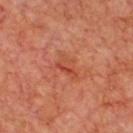The lesion was photographed on a routine skin check and not biopsied; there is no pathology result. Automated tile analysis of the lesion measured an area of roughly 4 mm², an outline eccentricity of about 0.85 (0 = round, 1 = elongated), and a symmetry-axis asymmetry near 0.45. The software also gave border irregularity of about 4 on a 0–10 scale, a color-variation rating of about 6.5/10, and peripheral color asymmetry of about 2.5. It also reported an automated nevus-likeness rating near 0 out of 100. The patient is a male in their mid- to late 60s. Imaged with cross-polarized lighting. The lesion is located on the chest. Approximately 3 mm at its widest. A region of skin cropped from a whole-body photographic capture, roughly 15 mm wide.